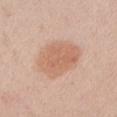Q: Was this lesion biopsied?
A: no biopsy performed (imaged during a skin exam)
Q: Patient demographics?
A: male, aged around 60
Q: Where on the body is the lesion?
A: the left upper arm
Q: What kind of image is this?
A: ~15 mm tile from a whole-body skin photo
Q: What lighting was used for the tile?
A: white-light